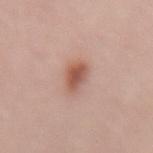This lesion was catalogued during total-body skin photography and was not selected for biopsy.
The lesion is located on the lower back.
The lesion's longest dimension is about 2.5 mm.
Captured under white-light illumination.
A 15 mm close-up extracted from a 3D total-body photography capture.
The subject is a female aged 38 to 42.